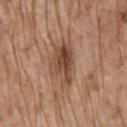Case summary:
- follow-up · no biopsy performed (imaged during a skin exam)
- lesion diameter · about 5.5 mm
- illumination · white-light
- location · the mid back
- imaging modality · ~15 mm tile from a whole-body skin photo
- subject · male, aged 68–72